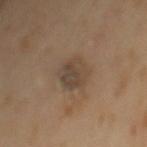Q: Is there a histopathology result?
A: catalogued during a skin exam; not biopsied
Q: Who is the patient?
A: female, aged 53 to 57
Q: Lesion location?
A: the back
Q: How was this image acquired?
A: total-body-photography crop, ~15 mm field of view